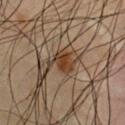biopsy status = no biopsy performed (imaged during a skin exam)
patient = male, aged approximately 60
automated lesion analysis = a lesion area of about 6.5 mm², a shape eccentricity near 0.55, and a symmetry-axis asymmetry near 0.45; an average lesion color of about L≈31 a*≈14 b*≈25 (CIELAB), about 8 CIELAB-L* units darker than the surrounding skin, and a normalized lesion–skin contrast near 9; border irregularity of about 5.5 on a 0–10 scale and peripheral color asymmetry of about 1
illumination = cross-polarized illumination
imaging modality = ~15 mm crop, total-body skin-cancer survey
anatomic site = the chest